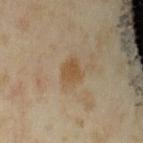Impression: Imaged during a routine full-body skin examination; the lesion was not biopsied and no histopathology is available. Clinical summary: The lesion is on the left upper arm. This image is a 15 mm lesion crop taken from a total-body photograph. The subject is a female about 35 years old.